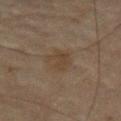<case>
<biopsy_status>not biopsied; imaged during a skin examination</biopsy_status>
<patient>
  <sex>male</sex>
  <age_approx>70</age_approx>
</patient>
<lighting>cross-polarized</lighting>
<site>abdomen</site>
<lesion_size>
  <long_diameter_mm_approx>3.0</long_diameter_mm_approx>
</lesion_size>
<image>
  <source>total-body photography crop</source>
  <field_of_view_mm>15</field_of_view_mm>
</image>
</case>